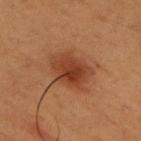follow-up — no biopsy performed (imaged during a skin exam); illumination — cross-polarized; site — the chest; subject — male, in their 50s; lesion size — about 5 mm; automated metrics — an eccentricity of roughly 0.7; imaging modality — ~15 mm tile from a whole-body skin photo.